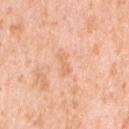Imaged during a routine full-body skin examination; the lesion was not biopsied and no histopathology is available. The lesion is on the right upper arm. The recorded lesion diameter is about 2.5 mm. A female subject in their 40s. Cropped from a whole-body photographic skin survey; the tile spans about 15 mm.